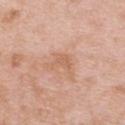workup = catalogued during a skin exam; not biopsied | anatomic site = the upper back | acquisition = 15 mm crop, total-body photography | subject = female, aged 63–67.